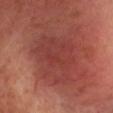Q: Is there a histopathology result?
A: catalogued during a skin exam; not biopsied
Q: What kind of image is this?
A: total-body-photography crop, ~15 mm field of view
Q: What are the patient's age and sex?
A: female, in their mid-50s
Q: Illumination type?
A: cross-polarized
Q: What is the lesion's diameter?
A: ≈10.5 mm
Q: Where on the body is the lesion?
A: the head or neck